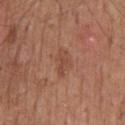Case summary:
- follow-up: imaged on a skin check; not biopsied
- illumination: white-light illumination
- size: ≈3 mm
- subject: male, roughly 60 years of age
- location: the mid back
- image: ~15 mm tile from a whole-body skin photo